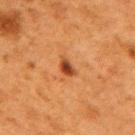{"biopsy_status": "not biopsied; imaged during a skin examination", "lesion_size": {"long_diameter_mm_approx": 2.5}, "patient": {"sex": "female", "age_approx": 50}, "image": {"source": "total-body photography crop", "field_of_view_mm": 15}, "automated_metrics": {"area_mm2_approx": 3.0, "eccentricity": 0.8, "shape_asymmetry": 0.35, "vs_skin_darker_L": 13.0, "vs_skin_contrast_norm": 10.0, "border_irregularity_0_10": 3.0, "color_variation_0_10": 3.0, "peripheral_color_asymmetry": 0.5, "nevus_likeness_0_100": 90, "lesion_detection_confidence_0_100": 100}, "site": "upper back"}